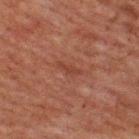{"biopsy_status": "not biopsied; imaged during a skin examination", "lighting": "cross-polarized", "image": {"source": "total-body photography crop", "field_of_view_mm": 15}, "site": "upper back", "patient": {"sex": "male", "age_approx": 60}, "lesion_size": {"long_diameter_mm_approx": 3.0}}